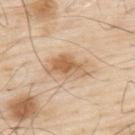Findings:
* workup — total-body-photography surveillance lesion; no biopsy
* location — the upper back
* automated metrics — a lesion area of about 10 mm² and a symmetry-axis asymmetry near 0.3; an average lesion color of about L≈63 a*≈18 b*≈36 (CIELAB), a lesion–skin lightness drop of about 11, and a normalized lesion–skin contrast near 7.5; internal color variation of about 5.5 on a 0–10 scale
* image source — ~15 mm crop, total-body skin-cancer survey
* lesion size — ~5 mm (longest diameter)
* patient — male, aged 78 to 82